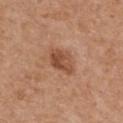Assessment: No biopsy was performed on this lesion — it was imaged during a full skin examination and was not determined to be concerning. Background: The tile uses white-light illumination. On the mid back. The patient is a male roughly 70 years of age. A roughly 15 mm field-of-view crop from a total-body skin photograph. Longest diameter approximately 4 mm.